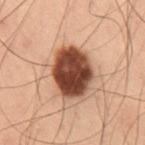<tbp_lesion>
  <automated_metrics>
    <cielab_L>35</cielab_L>
    <cielab_a>20</cielab_a>
    <cielab_b>25</cielab_b>
    <vs_skin_contrast_norm>16.0</vs_skin_contrast_norm>
    <border_irregularity_0_10>1.0</border_irregularity_0_10>
    <peripheral_color_asymmetry>1.5</peripheral_color_asymmetry>
    <nevus_likeness_0_100>55</nevus_likeness_0_100>
  </automated_metrics>
  <image>
    <source>total-body photography crop</source>
    <field_of_view_mm>15</field_of_view_mm>
  </image>
  <lighting>cross-polarized</lighting>
  <patient>
    <sex>male</sex>
    <age_approx>55</age_approx>
  </patient>
  <lesion_size>
    <long_diameter_mm_approx>5.5</long_diameter_mm_approx>
  </lesion_size>
  <site>right thigh</site>
</tbp_lesion>